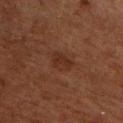The lesion was tiled from a total-body skin photograph and was not biopsied. From the front of the torso. Cropped from a whole-body photographic skin survey; the tile spans about 15 mm. Imaged with cross-polarized lighting. A male subject, roughly 60 years of age. Approximately 3.5 mm at its widest.